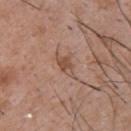Imaged during a routine full-body skin examination; the lesion was not biopsied and no histopathology is available. From the back. A 15 mm close-up tile from a total-body photography series done for melanoma screening. A male subject aged around 50. Captured under white-light illumination. Approximately 2.5 mm at its widest.